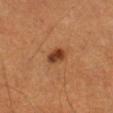Impression: No biopsy was performed on this lesion — it was imaged during a full skin examination and was not determined to be concerning. Background: The subject is a male aged approximately 60. A lesion tile, about 15 mm wide, cut from a 3D total-body photograph. The tile uses cross-polarized illumination. The lesion is on the right thigh.